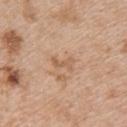{"biopsy_status": "not biopsied; imaged during a skin examination", "lighting": "white-light", "automated_metrics": {"area_mm2_approx": 3.5, "eccentricity": 0.85, "shape_asymmetry": 0.45, "cielab_L": 59, "cielab_a": 19, "cielab_b": 33, "vs_skin_contrast_norm": 5.5}, "image": {"source": "total-body photography crop", "field_of_view_mm": 15}, "site": "upper back", "patient": {"sex": "female", "age_approx": 45}}